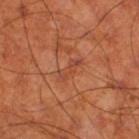<record>
  <biopsy_status>not biopsied; imaged during a skin examination</biopsy_status>
  <lighting>cross-polarized</lighting>
  <image>
    <source>total-body photography crop</source>
    <field_of_view_mm>15</field_of_view_mm>
  </image>
  <lesion_size>
    <long_diameter_mm_approx>3.5</long_diameter_mm_approx>
  </lesion_size>
  <patient>
    <sex>male</sex>
    <age_approx>70</age_approx>
  </patient>
  <automated_metrics>
    <area_mm2_approx>4.0</area_mm2_approx>
    <eccentricity>0.9</eccentricity>
    <shape_asymmetry>0.3</shape_asymmetry>
  </automated_metrics>
  <site>right thigh</site>
</record>